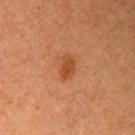Imaged during a routine full-body skin examination; the lesion was not biopsied and no histopathology is available. Imaged with cross-polarized lighting. A 15 mm crop from a total-body photograph taken for skin-cancer surveillance. Measured at roughly 3 mm in maximum diameter. A female patient, in their 60s. Automated image analysis of the tile measured an area of roughly 4 mm², a shape eccentricity near 0.75, and a shape-asymmetry score of about 0.25 (0 = symmetric). The software also gave a lesion color around L≈42 a*≈25 b*≈37 in CIELAB, a lesion–skin lightness drop of about 8, and a lesion-to-skin contrast of about 7.5 (normalized; higher = more distinct). It also reported a lesion-detection confidence of about 100/100. From the arm.